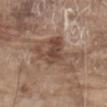The lesion was photographed on a routine skin check and not biopsied; there is no pathology result. On the front of the torso. A lesion tile, about 15 mm wide, cut from a 3D total-body photograph. The lesion's longest dimension is about 7.5 mm. A male patient, roughly 80 years of age.